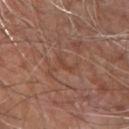Cropped from a total-body skin-imaging series; the visible field is about 15 mm.
From the right forearm.
A male patient about 80 years old.